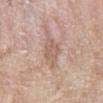Imaged during a routine full-body skin examination; the lesion was not biopsied and no histopathology is available. Located on the leg. A lesion tile, about 15 mm wide, cut from a 3D total-body photograph. The tile uses white-light illumination. A female subject, roughly 60 years of age.